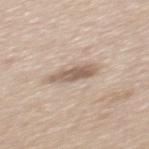Clinical impression:
No biopsy was performed on this lesion — it was imaged during a full skin examination and was not determined to be concerning.
Background:
On the back. A 15 mm crop from a total-body photograph taken for skin-cancer surveillance. Imaged with white-light lighting. The patient is a male roughly 60 years of age. The recorded lesion diameter is about 4.5 mm. An algorithmic analysis of the crop reported an area of roughly 6 mm² and a shape-asymmetry score of about 0.25 (0 = symmetric). The software also gave a lesion–skin lightness drop of about 13 and a normalized border contrast of about 8.